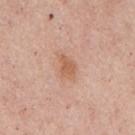workup=imaged on a skin check; not biopsied
imaging modality=~15 mm crop, total-body skin-cancer survey
lighting=white-light
subject=male, aged 53–57
lesion diameter=about 3 mm
site=the back